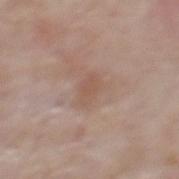Recorded during total-body skin imaging; not selected for excision or biopsy.
The subject is a male approximately 60 years of age.
The lesion is located on the back.
A 15 mm crop from a total-body photograph taken for skin-cancer surveillance.
Imaged with white-light lighting.